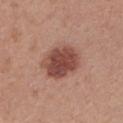Q: Was a biopsy performed?
A: no biopsy performed (imaged during a skin exam)
Q: Lesion location?
A: the chest
Q: What are the patient's age and sex?
A: female, roughly 25 years of age
Q: Automated lesion metrics?
A: a footprint of about 18 mm², an eccentricity of roughly 0.55, and a symmetry-axis asymmetry near 0.1; an automated nevus-likeness rating near 95 out of 100 and lesion-presence confidence of about 100/100
Q: How was the tile lit?
A: white-light illumination
Q: How was this image acquired?
A: 15 mm crop, total-body photography
Q: How large is the lesion?
A: about 5.5 mm